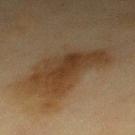Recorded during total-body skin imaging; not selected for excision or biopsy. The subject is a female approximately 40 years of age. Cropped from a total-body skin-imaging series; the visible field is about 15 mm. Imaged with cross-polarized lighting. On the upper back.